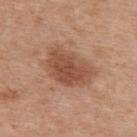Impression: The lesion was photographed on a routine skin check and not biopsied; there is no pathology result. Context: Measured at roughly 5.5 mm in maximum diameter. The lesion is on the upper back. The patient is a female roughly 40 years of age. An algorithmic analysis of the crop reported an average lesion color of about L≈51 a*≈22 b*≈31 (CIELAB), about 11 CIELAB-L* units darker than the surrounding skin, and a normalized border contrast of about 7.5. The software also gave a classifier nevus-likeness of about 75/100. Cropped from a total-body skin-imaging series; the visible field is about 15 mm.